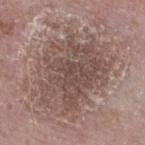image source = ~15 mm tile from a whole-body skin photo
body site = the right thigh
size = about 9.5 mm
tile lighting = white-light
subject = male, in their mid-70s